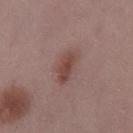biopsy status: imaged on a skin check; not biopsied
patient: female, aged around 30
acquisition: ~15 mm crop, total-body skin-cancer survey
body site: the right thigh
lesion size: ~4.5 mm (longest diameter)